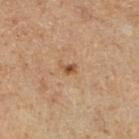{
  "image": {
    "source": "total-body photography crop",
    "field_of_view_mm": 15
  },
  "lighting": "cross-polarized",
  "patient": {
    "sex": "male",
    "age_approx": 55
  },
  "site": "right lower leg"
}